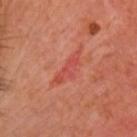Clinical impression:
This lesion was catalogued during total-body skin photography and was not selected for biopsy.
Clinical summary:
Captured under cross-polarized illumination. A female patient in their 50s. A 15 mm close-up tile from a total-body photography series done for melanoma screening. Measured at roughly 5.5 mm in maximum diameter. Automated tile analysis of the lesion measured a lesion color around L≈51 a*≈35 b*≈32 in CIELAB, about 7 CIELAB-L* units darker than the surrounding skin, and a normalized border contrast of about 4.5. It also reported a border-irregularity rating of about 5.5/10 and peripheral color asymmetry of about 1. It also reported an automated nevus-likeness rating near 0 out of 100 and lesion-presence confidence of about 80/100.